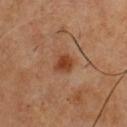Q: Was a biopsy performed?
A: catalogued during a skin exam; not biopsied
Q: Lesion location?
A: the chest
Q: Illumination type?
A: cross-polarized
Q: What are the patient's age and sex?
A: male, in their 50s
Q: Lesion size?
A: ≈2.5 mm
Q: How was this image acquired?
A: ~15 mm tile from a whole-body skin photo
Q: Automated lesion metrics?
A: an average lesion color of about L≈42 a*≈25 b*≈34 (CIELAB), about 10 CIELAB-L* units darker than the surrounding skin, and a normalized border contrast of about 9; a classifier nevus-likeness of about 95/100 and a lesion-detection confidence of about 100/100